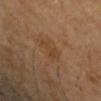No biopsy was performed on this lesion — it was imaged during a full skin examination and was not determined to be concerning. A close-up tile cropped from a whole-body skin photograph, about 15 mm across. An algorithmic analysis of the crop reported an area of roughly 5 mm² and two-axis asymmetry of about 0.45. From the arm. The recorded lesion diameter is about 3 mm. Imaged with cross-polarized lighting. The patient is a female aged around 60.